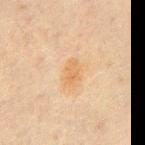{
  "site": "mid back",
  "lighting": "cross-polarized",
  "lesion_size": {
    "long_diameter_mm_approx": 3.0
  },
  "patient": {
    "sex": "male",
    "age_approx": 70
  },
  "image": {
    "source": "total-body photography crop",
    "field_of_view_mm": 15
  }
}